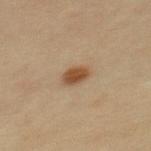Imaged during a routine full-body skin examination; the lesion was not biopsied and no histopathology is available. The lesion is on the mid back. An algorithmic analysis of the crop reported a color-variation rating of about 2.5/10. The analysis additionally found a nevus-likeness score of about 100/100. This is a cross-polarized tile. A male patient, aged 38–42. A lesion tile, about 15 mm wide, cut from a 3D total-body photograph.